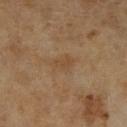This lesion was catalogued during total-body skin photography and was not selected for biopsy. A region of skin cropped from a whole-body photographic capture, roughly 15 mm wide. A male patient, roughly 65 years of age. From the right lower leg.